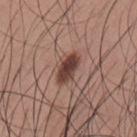Recorded during total-body skin imaging; not selected for excision or biopsy.
About 4 mm across.
Automated tile analysis of the lesion measured a nevus-likeness score of about 95/100.
The lesion is on the back.
A male patient, in their mid-30s.
Imaged with white-light lighting.
A close-up tile cropped from a whole-body skin photograph, about 15 mm across.